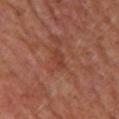Imaged during a routine full-body skin examination; the lesion was not biopsied and no histopathology is available.
The tile uses cross-polarized illumination.
Automated tile analysis of the lesion measured a border-irregularity index near 2/10, internal color variation of about 0.5 on a 0–10 scale, and radial color variation of about 0. The software also gave a classifier nevus-likeness of about 0/100 and a detector confidence of about 100 out of 100 that the crop contains a lesion.
A female subject approximately 60 years of age.
The lesion is located on the upper back.
About 2.5 mm across.
Cropped from a whole-body photographic skin survey; the tile spans about 15 mm.